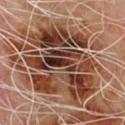- notes — no biopsy performed (imaged during a skin exam)
- subject — male, aged 63–67
- image — ~15 mm crop, total-body skin-cancer survey
- tile lighting — cross-polarized
- anatomic site — the chest
- size — about 11 mm